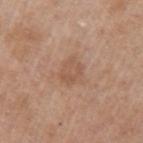biopsy status — catalogued during a skin exam; not biopsied
automated metrics — an average lesion color of about L≈54 a*≈20 b*≈31 (CIELAB), roughly 7 lightness units darker than nearby skin, and a normalized border contrast of about 5; border irregularity of about 6 on a 0–10 scale, a color-variation rating of about 0.5/10, and peripheral color asymmetry of about 0; a classifier nevus-likeness of about 0/100
location — the left upper arm
subject — male, in their 60s
size — ≈2.5 mm
imaging modality — 15 mm crop, total-body photography